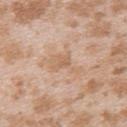biopsy status: no biopsy performed (imaged during a skin exam) | tile lighting: white-light illumination | size: about 4 mm | location: the upper back | image: ~15 mm tile from a whole-body skin photo | subject: female, aged 23–27.